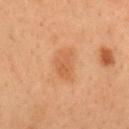Clinical impression: This lesion was catalogued during total-body skin photography and was not selected for biopsy. Context: From the front of the torso. Cropped from a whole-body photographic skin survey; the tile spans about 15 mm. A male patient aged approximately 55. About 3.5 mm across. An algorithmic analysis of the crop reported a lesion area of about 6.5 mm², an eccentricity of roughly 0.75, and two-axis asymmetry of about 0.25. And it measured a mean CIELAB color near L≈48 a*≈21 b*≈33 and a normalized lesion–skin contrast near 5.5. It also reported lesion-presence confidence of about 100/100.